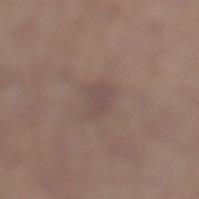biopsy_status: not biopsied; imaged during a skin examination
image:
  source: total-body photography crop
  field_of_view_mm: 15
lesion_size:
  long_diameter_mm_approx: 2.5
site: left lower leg
patient:
  sex: male
  age_approx: 55